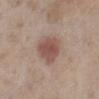The lesion was tiled from a total-body skin photograph and was not biopsied. A male subject, aged around 60. The lesion is located on the leg. A 15 mm close-up extracted from a 3D total-body photography capture.